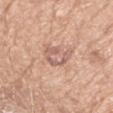<tbp_lesion>
  <biopsy_status>not biopsied; imaged during a skin examination</biopsy_status>
  <site>arm</site>
  <image>
    <source>total-body photography crop</source>
    <field_of_view_mm>15</field_of_view_mm>
  </image>
  <patient>
    <sex>female</sex>
    <age_approx>75</age_approx>
  </patient>
</tbp_lesion>